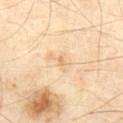No biopsy was performed on this lesion — it was imaged during a full skin examination and was not determined to be concerning.
From the abdomen.
The lesion-visualizer software estimated a lesion color around L≈71 a*≈18 b*≈38 in CIELAB, a lesion–skin lightness drop of about 8, and a normalized lesion–skin contrast near 5. It also reported a nevus-likeness score of about 0/100 and lesion-presence confidence of about 100/100.
Imaged with cross-polarized lighting.
A 15 mm crop from a total-body photograph taken for skin-cancer surveillance.
The patient is a male roughly 70 years of age.